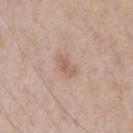Assessment:
This lesion was catalogued during total-body skin photography and was not selected for biopsy.
Image and clinical context:
The total-body-photography lesion software estimated an area of roughly 4 mm², a shape eccentricity near 0.85, and a symmetry-axis asymmetry near 0.2. The analysis additionally found a border-irregularity rating of about 2.5/10, internal color variation of about 2.5 on a 0–10 scale, and a peripheral color-asymmetry measure near 1. About 3 mm across. A male subject, in their mid-60s. The lesion is located on the chest. A lesion tile, about 15 mm wide, cut from a 3D total-body photograph. The tile uses white-light illumination.